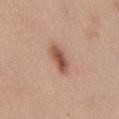Q: Was this lesion biopsied?
A: catalogued during a skin exam; not biopsied
Q: What is the anatomic site?
A: the front of the torso
Q: What lighting was used for the tile?
A: white-light
Q: Lesion size?
A: ≈4 mm
Q: How was this image acquired?
A: ~15 mm tile from a whole-body skin photo
Q: Who is the patient?
A: male, roughly 30 years of age